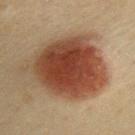No biopsy was performed on this lesion — it was imaged during a full skin examination and was not determined to be concerning.
Measured at roughly 9 mm in maximum diameter.
Captured under cross-polarized illumination.
A close-up tile cropped from a whole-body skin photograph, about 15 mm across.
The patient is a male aged approximately 40.
The lesion is on the upper back.